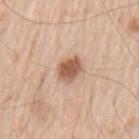Clinical impression:
Recorded during total-body skin imaging; not selected for excision or biopsy.
Clinical summary:
A lesion tile, about 15 mm wide, cut from a 3D total-body photograph. A male subject aged 53–57. Longest diameter approximately 3 mm. Imaged with white-light lighting. Located on the left upper arm. Automated tile analysis of the lesion measured border irregularity of about 2 on a 0–10 scale and radial color variation of about 0.5.